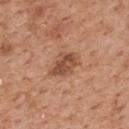follow-up = no biopsy performed (imaged during a skin exam); subject = male, aged 58–62; acquisition = total-body-photography crop, ~15 mm field of view; lesion diameter = ≈4 mm; automated lesion analysis = an automated nevus-likeness rating near 55 out of 100 and lesion-presence confidence of about 100/100; illumination = white-light; body site = the back.